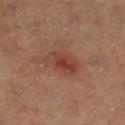Q: Was this lesion biopsied?
A: imaged on a skin check; not biopsied